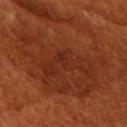Impression: Part of a total-body skin-imaging series; this lesion was reviewed on a skin check and was not flagged for biopsy. Context: The total-body-photography lesion software estimated border irregularity of about 5.5 on a 0–10 scale and a within-lesion color-variation index near 3/10. And it measured a classifier nevus-likeness of about 0/100. The patient is a female aged 78–82. A lesion tile, about 15 mm wide, cut from a 3D total-body photograph. Located on the head or neck. Imaged with cross-polarized lighting.